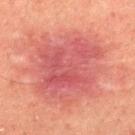Clinical impression:
The lesion was photographed on a routine skin check and not biopsied; there is no pathology result.